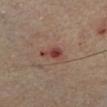Impression:
Captured during whole-body skin photography for melanoma surveillance; the lesion was not biopsied.
Clinical summary:
A male subject approximately 65 years of age. Longest diameter approximately 3 mm. This image is a 15 mm lesion crop taken from a total-body photograph. Automated tile analysis of the lesion measured a lesion area of about 3.5 mm², a shape eccentricity near 0.85, and a symmetry-axis asymmetry near 0.5. The analysis additionally found border irregularity of about 4.5 on a 0–10 scale, a color-variation rating of about 2.5/10, and a peripheral color-asymmetry measure near 0.5. The tile uses cross-polarized illumination. From the leg.